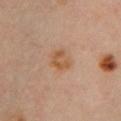<lesion>
<biopsy_status>not biopsied; imaged during a skin examination</biopsy_status>
<site>chest</site>
<lesion_size>
  <long_diameter_mm_approx>3.0</long_diameter_mm_approx>
</lesion_size>
<lighting>cross-polarized</lighting>
<image>
  <source>total-body photography crop</source>
  <field_of_view_mm>15</field_of_view_mm>
</image>
<patient>
  <sex>female</sex>
  <age_approx>35</age_approx>
</patient>
</lesion>